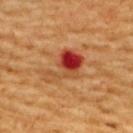<record>
<biopsy_status>not biopsied; imaged during a skin examination</biopsy_status>
<image>
  <source>total-body photography crop</source>
  <field_of_view_mm>15</field_of_view_mm>
</image>
<lesion_size>
  <long_diameter_mm_approx>5.0</long_diameter_mm_approx>
</lesion_size>
<patient>
  <sex>male</sex>
  <age_approx>60</age_approx>
</patient>
<site>upper back</site>
</record>